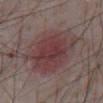follow-up — imaged on a skin check; not biopsied | lesion size — ≈8 mm | subject — male, in their mid-70s | site — the abdomen | TBP lesion metrics — a footprint of about 35 mm²; a border-irregularity index near 2/10 and peripheral color asymmetry of about 1.5 | tile lighting — white-light illumination | image — total-body-photography crop, ~15 mm field of view.